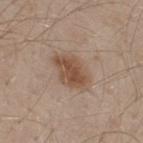Part of a total-body skin-imaging series; this lesion was reviewed on a skin check and was not flagged for biopsy.
A region of skin cropped from a whole-body photographic capture, roughly 15 mm wide.
A male subject roughly 30 years of age.
From the back.